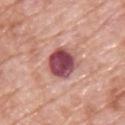No biopsy was performed on this lesion — it was imaged during a full skin examination and was not determined to be concerning. The lesion is located on the front of the torso. Automated image analysis of the tile measured an area of roughly 11 mm², a shape eccentricity near 0.7, and a shape-asymmetry score of about 0.15 (0 = symmetric). The analysis additionally found a mean CIELAB color near L≈48 a*≈29 b*≈19 and about 19 CIELAB-L* units darker than the surrounding skin. A male subject, about 70 years old. About 4.5 mm across. Cropped from a total-body skin-imaging series; the visible field is about 15 mm. This is a white-light tile.